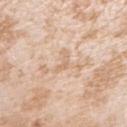Background:
A lesion tile, about 15 mm wide, cut from a 3D total-body photograph. Measured at roughly 3 mm in maximum diameter. On the right upper arm. Imaged with white-light lighting. A female subject, about 25 years old.